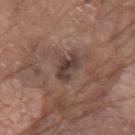Recorded during total-body skin imaging; not selected for excision or biopsy. Cropped from a total-body skin-imaging series; the visible field is about 15 mm. Imaged with white-light lighting. The patient is a male aged around 80. An algorithmic analysis of the crop reported border irregularity of about 3.5 on a 0–10 scale and a peripheral color-asymmetry measure near 1. The lesion is on the left upper arm.